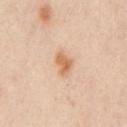Q: Was a biopsy performed?
A: total-body-photography surveillance lesion; no biopsy
Q: Lesion size?
A: about 3 mm
Q: What are the patient's age and sex?
A: aged 53 to 57
Q: Illumination type?
A: cross-polarized illumination
Q: Where on the body is the lesion?
A: the chest
Q: What is the imaging modality?
A: ~15 mm crop, total-body skin-cancer survey